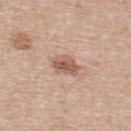<case>
  <biopsy_status>not biopsied; imaged during a skin examination</biopsy_status>
  <image>
    <source>total-body photography crop</source>
    <field_of_view_mm>15</field_of_view_mm>
  </image>
  <patient>
    <sex>female</sex>
    <age_approx>40</age_approx>
  </patient>
  <site>upper back</site>
  <lesion_size>
    <long_diameter_mm_approx>3.5</long_diameter_mm_approx>
  </lesion_size>
  <lighting>white-light</lighting>
</case>